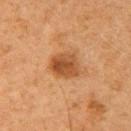notes = catalogued during a skin exam; not biopsied | imaging modality = total-body-photography crop, ~15 mm field of view | tile lighting = cross-polarized | anatomic site = the right upper arm | automated metrics = border irregularity of about 2 on a 0–10 scale, a color-variation rating of about 4/10, and radial color variation of about 1.5; a classifier nevus-likeness of about 75/100 and lesion-presence confidence of about 100/100 | lesion size = ≈3.5 mm | subject = male, roughly 65 years of age.